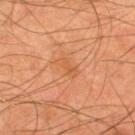A roughly 15 mm field-of-view crop from a total-body skin photograph.
A male patient, roughly 45 years of age.
The lesion's longest dimension is about 3 mm.
From the back.
This is a cross-polarized tile.